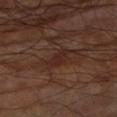Notes:
• biopsy status: no biopsy performed (imaged during a skin exam)